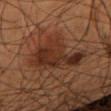biopsy_status: not biopsied; imaged during a skin examination
patient:
  sex: male
  age_approx: 55
lighting: cross-polarized
automated_metrics:
  border_irregularity_0_10: 6.0
  color_variation_0_10: 4.5
  peripheral_color_asymmetry: 1.5
image:
  source: total-body photography crop
  field_of_view_mm: 15
lesion_size:
  long_diameter_mm_approx: 7.5
site: right forearm